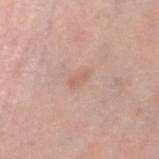Notes:
- notes: no biopsy performed (imaged during a skin exam)
- acquisition: ~15 mm crop, total-body skin-cancer survey
- site: the left lower leg
- tile lighting: white-light
- patient: female, aged around 40
- automated lesion analysis: a footprint of about 3 mm², an outline eccentricity of about 0.85 (0 = round, 1 = elongated), and a symmetry-axis asymmetry near 0.35; a border-irregularity index near 3.5/10, internal color variation of about 0.5 on a 0–10 scale, and a peripheral color-asymmetry measure near 0; a nevus-likeness score of about 0/100 and a detector confidence of about 100 out of 100 that the crop contains a lesion